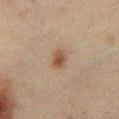The lesion was photographed on a routine skin check and not biopsied; there is no pathology result. Located on the chest. A close-up tile cropped from a whole-body skin photograph, about 15 mm across. The recorded lesion diameter is about 3 mm. A male patient, roughly 50 years of age. Captured under cross-polarized illumination.